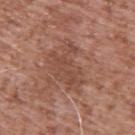  biopsy_status: not biopsied; imaged during a skin examination
  patient:
    sex: male
    age_approx: 55
  image:
    source: total-body photography crop
    field_of_view_mm: 15
  automated_metrics:
    area_mm2_approx: 14.0
    eccentricity: 0.8
    cielab_L: 47
    cielab_a: 22
    cielab_b: 27
    vs_skin_darker_L: 7.0
    border_irregularity_0_10: 6.0
    color_variation_0_10: 2.5
    peripheral_color_asymmetry: 1.0
  lesion_size:
    long_diameter_mm_approx: 6.0
  site: upper back